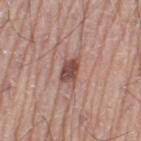Assessment: The lesion was tiled from a total-body skin photograph and was not biopsied. Acquisition and patient details: From the leg. An algorithmic analysis of the crop reported a footprint of about 5 mm² and an outline eccentricity of about 0.8 (0 = round, 1 = elongated). And it measured a lesion-detection confidence of about 100/100. Cropped from a total-body skin-imaging series; the visible field is about 15 mm. The subject is a male aged approximately 70. The recorded lesion diameter is about 3 mm.